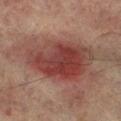image source = 15 mm crop, total-body photography
image-analysis metrics = a lesion area of about 33 mm², an eccentricity of roughly 0.75, and a symmetry-axis asymmetry near 0.2; a lesion color around L≈34 a*≈22 b*≈21 in CIELAB, a lesion–skin lightness drop of about 10, and a normalized lesion–skin contrast near 9.5
lighting = cross-polarized illumination
body site = the left lower leg
subject = male, roughly 75 years of age
lesion diameter = ≈8 mm